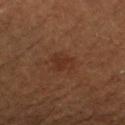Assessment: The lesion was photographed on a routine skin check and not biopsied; there is no pathology result. Context: Automated tile analysis of the lesion measured an outline eccentricity of about 0.55 (0 = round, 1 = elongated) and a shape-asymmetry score of about 0.25 (0 = symmetric). This image is a 15 mm lesion crop taken from a total-body photograph. The patient is a female aged around 80. From the right lower leg. About 3 mm across.